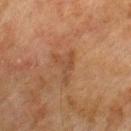No biopsy was performed on this lesion — it was imaged during a full skin examination and was not determined to be concerning.
The lesion is located on the arm.
The subject is a male aged 73 to 77.
A 15 mm close-up tile from a total-body photography series done for melanoma screening.
Imaged with cross-polarized lighting.
Longest diameter approximately 3.5 mm.